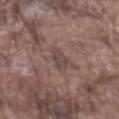{
  "biopsy_status": "not biopsied; imaged during a skin examination"
}